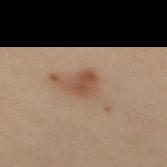follow-up = no biopsy performed (imaged during a skin exam) | location = the mid back | illumination = cross-polarized illumination | image-analysis metrics = an area of roughly 5.5 mm², a shape eccentricity near 0.6, and two-axis asymmetry of about 0.25; a mean CIELAB color near L≈39 a*≈16 b*≈24, about 8 CIELAB-L* units darker than the surrounding skin, and a normalized lesion–skin contrast near 7; internal color variation of about 3 on a 0–10 scale and radial color variation of about 1; an automated nevus-likeness rating near 60 out of 100 | imaging modality = total-body-photography crop, ~15 mm field of view | subject = female, aged 28–32 | diameter = about 3 mm.